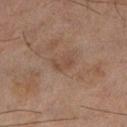• follow-up: total-body-photography surveillance lesion; no biopsy
• lighting: cross-polarized
• location: the left lower leg
• lesion diameter: about 2.5 mm
• patient: male, in their mid- to late 40s
• image-analysis metrics: an average lesion color of about L≈36 a*≈15 b*≈23 (CIELAB); a color-variation rating of about 0/10 and peripheral color asymmetry of about 0; an automated nevus-likeness rating near 0 out of 100
• imaging modality: ~15 mm crop, total-body skin-cancer survey